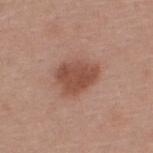Impression:
The lesion was tiled from a total-body skin photograph and was not biopsied.
Clinical summary:
A male subject about 40 years old. The tile uses white-light illumination. A 15 mm close-up tile from a total-body photography series done for melanoma screening. Located on the upper back.